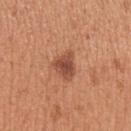lighting = white-light; subject = female, about 30 years old; site = the left upper arm; image source = ~15 mm tile from a whole-body skin photo.